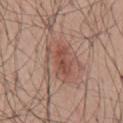Q: Was a biopsy performed?
A: total-body-photography surveillance lesion; no biopsy
Q: What is the imaging modality?
A: ~15 mm tile from a whole-body skin photo
Q: How large is the lesion?
A: ≈5.5 mm
Q: Lesion location?
A: the back
Q: What are the patient's age and sex?
A: male, approximately 55 years of age
Q: How was the tile lit?
A: white-light